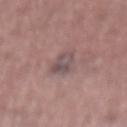Captured during whole-body skin photography for melanoma surveillance; the lesion was not biopsied. A male patient, aged approximately 60. Captured under white-light illumination. The lesion is located on the mid back. A 15 mm crop from a total-body photograph taken for skin-cancer surveillance. An algorithmic analysis of the crop reported an area of roughly 5.5 mm² and a shape-asymmetry score of about 0.3 (0 = symmetric). It also reported a border-irregularity rating of about 3/10, a color-variation rating of about 5/10, and peripheral color asymmetry of about 2. The software also gave a nevus-likeness score of about 0/100 and a detector confidence of about 90 out of 100 that the crop contains a lesion.